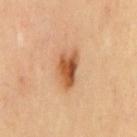Context: On the chest. The tile uses cross-polarized illumination. The lesion-visualizer software estimated a classifier nevus-likeness of about 100/100 and lesion-presence confidence of about 100/100. A male patient, aged 68–72. A 15 mm close-up extracted from a 3D total-body photography capture. Approximately 4.5 mm at its widest.